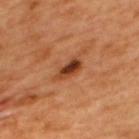{
  "biopsy_status": "not biopsied; imaged during a skin examination",
  "automated_metrics": {
    "shape_asymmetry": 0.3,
    "nevus_likeness_0_100": 70,
    "lesion_detection_confidence_0_100": 100
  },
  "site": "upper back",
  "patient": {
    "sex": "male",
    "age_approx": 50
  },
  "image": {
    "source": "total-body photography crop",
    "field_of_view_mm": 15
  },
  "lesion_size": {
    "long_diameter_mm_approx": 3.5
  }
}